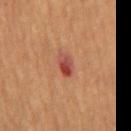Background:
Cropped from a whole-body photographic skin survey; the tile spans about 15 mm. This is a cross-polarized tile. A male subject aged 63 to 67. The lesion is on the abdomen.